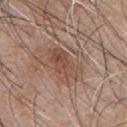Q: Is there a histopathology result?
A: no biopsy performed (imaged during a skin exam)
Q: Where on the body is the lesion?
A: the chest
Q: What did automated image analysis measure?
A: a mean CIELAB color near L≈48 a*≈19 b*≈27, roughly 8 lightness units darker than nearby skin, and a lesion-to-skin contrast of about 6.5 (normalized; higher = more distinct); border irregularity of about 4 on a 0–10 scale, internal color variation of about 5 on a 0–10 scale, and a peripheral color-asymmetry measure near 2
Q: How was this image acquired?
A: 15 mm crop, total-body photography
Q: How was the tile lit?
A: white-light illumination
Q: Lesion size?
A: about 4.5 mm
Q: What are the patient's age and sex?
A: male, roughly 45 years of age